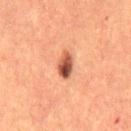Imaged during a routine full-body skin examination; the lesion was not biopsied and no histopathology is available.